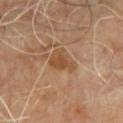Findings:
* notes: no biopsy performed (imaged during a skin exam)